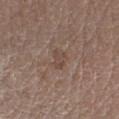Clinical impression: The lesion was tiled from a total-body skin photograph and was not biopsied. Image and clinical context: A close-up tile cropped from a whole-body skin photograph, about 15 mm across. This is a white-light tile. The lesion is located on the left forearm. The lesion-visualizer software estimated a lesion color around L≈45 a*≈16 b*≈24 in CIELAB, a lesion–skin lightness drop of about 6, and a lesion-to-skin contrast of about 5.5 (normalized; higher = more distinct). The software also gave a color-variation rating of about 1/10 and a peripheral color-asymmetry measure near 0. The analysis additionally found an automated nevus-likeness rating near 0 out of 100. A female patient, aged 48–52. The lesion's longest dimension is about 2.5 mm.